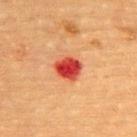This lesion was catalogued during total-body skin photography and was not selected for biopsy. About 3 mm across. Automated image analysis of the tile measured a mean CIELAB color near L≈42 a*≈41 b*≈35 and a normalized lesion–skin contrast near 12. And it measured a classifier nevus-likeness of about 0/100 and lesion-presence confidence of about 100/100. Imaged with cross-polarized lighting. A 15 mm close-up extracted from a 3D total-body photography capture. The lesion is on the upper back. The patient is a female about 70 years old.